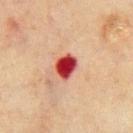notes = catalogued during a skin exam; not biopsied | tile lighting = cross-polarized illumination | patient = male, roughly 70 years of age | image = total-body-photography crop, ~15 mm field of view | lesion size = ~3 mm (longest diameter) | anatomic site = the chest.